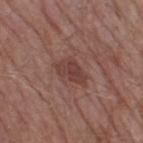<tbp_lesion>
  <biopsy_status>not biopsied; imaged during a skin examination</biopsy_status>
  <site>right thigh</site>
  <lesion_size>
    <long_diameter_mm_approx>4.0</long_diameter_mm_approx>
  </lesion_size>
  <automated_metrics>
    <area_mm2_approx>6.5</area_mm2_approx>
    <shape_asymmetry>0.25</shape_asymmetry>
    <cielab_L>40</cielab_L>
    <cielab_a>22</cielab_a>
    <cielab_b>22</cielab_b>
    <vs_skin_contrast_norm>7.0</vs_skin_contrast_norm>
    <border_irregularity_0_10>3.0</border_irregularity_0_10>
    <color_variation_0_10>3.0</color_variation_0_10>
    <peripheral_color_asymmetry>1.0</peripheral_color_asymmetry>
  </automated_metrics>
  <image>
    <source>total-body photography crop</source>
    <field_of_view_mm>15</field_of_view_mm>
  </image>
  <lighting>white-light</lighting>
  <patient>
    <sex>male</sex>
    <age_approx>55</age_approx>
  </patient>
</tbp_lesion>